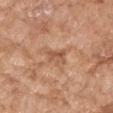The lesion was tiled from a total-body skin photograph and was not biopsied. The lesion's longest dimension is about 3 mm. A female subject about 75 years old. The lesion-visualizer software estimated an average lesion color of about L≈55 a*≈22 b*≈33 (CIELAB), a lesion–skin lightness drop of about 9, and a lesion-to-skin contrast of about 6 (normalized; higher = more distinct). The software also gave an automated nevus-likeness rating near 0 out of 100. This image is a 15 mm lesion crop taken from a total-body photograph. Imaged with white-light lighting. The lesion is located on the right forearm.